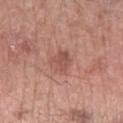A male subject aged approximately 60. Automated tile analysis of the lesion measured an eccentricity of roughly 0.5 and two-axis asymmetry of about 0.3. And it measured a classifier nevus-likeness of about 0/100 and lesion-presence confidence of about 100/100. A 15 mm close-up extracted from a 3D total-body photography capture. The tile uses white-light illumination. From the right forearm. Measured at roughly 3 mm in maximum diameter.